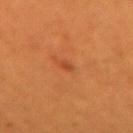Assessment: This lesion was catalogued during total-body skin photography and was not selected for biopsy. Context: Approximately 1.5 mm at its widest. The tile uses cross-polarized illumination. From the left forearm. The total-body-photography lesion software estimated an average lesion color of about L≈43 a*≈30 b*≈38 (CIELAB) and a lesion-to-skin contrast of about 6 (normalized; higher = more distinct). The software also gave a classifier nevus-likeness of about 5/100 and a detector confidence of about 100 out of 100 that the crop contains a lesion. A female subject aged approximately 30. A close-up tile cropped from a whole-body skin photograph, about 15 mm across.